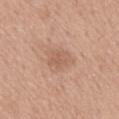Imaged during a routine full-body skin examination; the lesion was not biopsied and no histopathology is available. A male subject, about 60 years old. The lesion is located on the mid back. This is a white-light tile. A close-up tile cropped from a whole-body skin photograph, about 15 mm across.